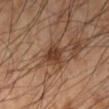Assessment: The lesion was tiled from a total-body skin photograph and was not biopsied. Clinical summary: Approximately 3.5 mm at its widest. The lesion is on the left lower leg. Automated image analysis of the tile measured a lesion color around L≈39 a*≈20 b*≈30 in CIELAB, roughly 11 lightness units darker than nearby skin, and a normalized border contrast of about 9. It also reported a classifier nevus-likeness of about 80/100 and a detector confidence of about 100 out of 100 that the crop contains a lesion. A male subject, in their 50s. Cropped from a total-body skin-imaging series; the visible field is about 15 mm. This is a cross-polarized tile.